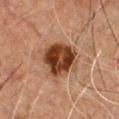biopsy_status: not biopsied; imaged during a skin examination
lesion_size:
  long_diameter_mm_approx: 4.5
patient:
  sex: male
  age_approx: 50
lighting: cross-polarized
site: chest
image:
  source: total-body photography crop
  field_of_view_mm: 15